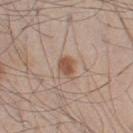This lesion was catalogued during total-body skin photography and was not selected for biopsy.
The total-body-photography lesion software estimated a shape-asymmetry score of about 0.25 (0 = symmetric). And it measured an average lesion color of about L≈53 a*≈19 b*≈29 (CIELAB), a lesion–skin lightness drop of about 11, and a lesion-to-skin contrast of about 8.5 (normalized; higher = more distinct). And it measured a nevus-likeness score of about 90/100 and lesion-presence confidence of about 100/100.
This image is a 15 mm lesion crop taken from a total-body photograph.
The subject is a male in their mid- to late 40s.
Approximately 2.5 mm at its widest.
The lesion is located on the left thigh.
The tile uses white-light illumination.